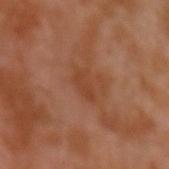A male subject roughly 30 years of age. The lesion is located on the left upper arm. A roughly 15 mm field-of-view crop from a total-body skin photograph. Imaged with cross-polarized lighting. The total-body-photography lesion software estimated a lesion color around L≈41 a*≈24 b*≈32 in CIELAB, a lesion–skin lightness drop of about 6, and a normalized border contrast of about 5. The analysis additionally found an automated nevus-likeness rating near 0 out of 100 and a detector confidence of about 100 out of 100 that the crop contains a lesion.